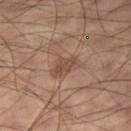notes = total-body-photography surveillance lesion; no biopsy.